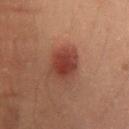Notes:
- notes · catalogued during a skin exam; not biopsied
- image source · 15 mm crop, total-body photography
- lighting · cross-polarized
- patient · male, aged 48–52
- anatomic site · the lower back
- lesion size · ~4 mm (longest diameter)